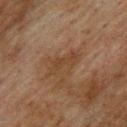site: the back | patient: male, aged 73 to 77 | image source: ~15 mm tile from a whole-body skin photo | tile lighting: cross-polarized illumination | image-analysis metrics: a lesion area of about 6 mm² and a symmetry-axis asymmetry near 0.45; roughly 6 lightness units darker than nearby skin and a normalized lesion–skin contrast near 6.